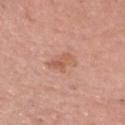Findings:
* lighting · white-light
* image-analysis metrics · a lesion area of about 6 mm² and a shape-asymmetry score of about 0.3 (0 = symmetric)
* imaging modality · 15 mm crop, total-body photography
* patient · male, aged 78–82
* anatomic site · the head or neck
* lesion diameter · ~3.5 mm (longest diameter)